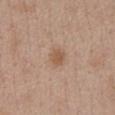* workup · catalogued during a skin exam; not biopsied
* site · the chest
* imaging modality · ~15 mm tile from a whole-body skin photo
* patient · female, aged 38 to 42
* lighting · white-light
* lesion size · about 2.5 mm
* image-analysis metrics · a lesion area of about 3.5 mm², a shape eccentricity near 0.65, and a shape-asymmetry score of about 0.25 (0 = symmetric); a lesion color around L≈55 a*≈19 b*≈31 in CIELAB, about 8 CIELAB-L* units darker than the surrounding skin, and a normalized border contrast of about 6.5; an automated nevus-likeness rating near 50 out of 100 and lesion-presence confidence of about 100/100